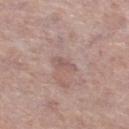Imaged during a routine full-body skin examination; the lesion was not biopsied and no histopathology is available.
A female subject, aged 58–62.
Located on the left lower leg.
Measured at roughly 2.5 mm in maximum diameter.
A roughly 15 mm field-of-view crop from a total-body skin photograph.
Imaged with white-light lighting.